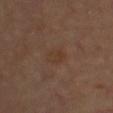Findings:
– biopsy status · catalogued during a skin exam; not biopsied
– image · ~15 mm tile from a whole-body skin photo
– illumination · cross-polarized
– patient · male, roughly 65 years of age
– body site · the front of the torso